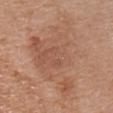{"biopsy_status": "not biopsied; imaged during a skin examination", "lesion_size": {"long_diameter_mm_approx": 10.0}, "lighting": "white-light", "automated_metrics": {"area_mm2_approx": 44.0, "eccentricity": 0.65, "shape_asymmetry": 0.5, "border_irregularity_0_10": 7.0, "color_variation_0_10": 5.0, "peripheral_color_asymmetry": 1.5}, "site": "chest", "patient": {"sex": "female", "age_approx": 65}, "image": {"source": "total-body photography crop", "field_of_view_mm": 15}}